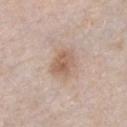This image is a 15 mm lesion crop taken from a total-body photograph.
From the chest.
A male subject, aged approximately 40.